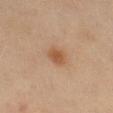This lesion was catalogued during total-body skin photography and was not selected for biopsy.
From the left thigh.
A close-up tile cropped from a whole-body skin photograph, about 15 mm across.
The total-body-photography lesion software estimated two-axis asymmetry of about 0.2. It also reported a lesion color around L≈48 a*≈19 b*≈32 in CIELAB and a normalized lesion–skin contrast near 7.5. It also reported a border-irregularity rating of about 1.5/10, a color-variation rating of about 2/10, and radial color variation of about 0.5.
Measured at roughly 2.5 mm in maximum diameter.
A female patient aged approximately 60.
The tile uses cross-polarized illumination.